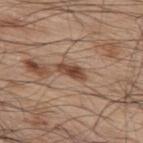Assessment: Imaged during a routine full-body skin examination; the lesion was not biopsied and no histopathology is available. Clinical summary: A male subject aged 68 to 72. The recorded lesion diameter is about 3 mm. A lesion tile, about 15 mm wide, cut from a 3D total-body photograph. The total-body-photography lesion software estimated an average lesion color of about L≈44 a*≈20 b*≈29 (CIELAB) and a lesion-to-skin contrast of about 10.5 (normalized; higher = more distinct). From the upper back. This is a white-light tile.